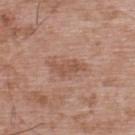<lesion>
  <biopsy_status>not biopsied; imaged during a skin examination</biopsy_status>
  <patient>
    <sex>male</sex>
    <age_approx>50</age_approx>
  </patient>
  <image>
    <source>total-body photography crop</source>
    <field_of_view_mm>15</field_of_view_mm>
  </image>
  <site>upper back</site>
</lesion>